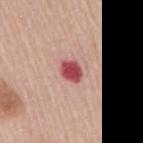<lesion>
<biopsy_status>not biopsied; imaged during a skin examination</biopsy_status>
<patient>
  <sex>female</sex>
  <age_approx>65</age_approx>
</patient>
<image>
  <source>total-body photography crop</source>
  <field_of_view_mm>15</field_of_view_mm>
</image>
<lesion_size>
  <long_diameter_mm_approx>3.0</long_diameter_mm_approx>
</lesion_size>
<automated_metrics>
  <eccentricity>0.7</eccentricity>
  <shape_asymmetry>0.2</shape_asymmetry>
  <cielab_L>52</cielab_L>
  <cielab_a>35</cielab_a>
  <cielab_b>23</cielab_b>
  <vs_skin_darker_L>17.0</vs_skin_darker_L>
  <nevus_likeness_0_100>0</nevus_likeness_0_100>
</automated_metrics>
<site>mid back</site>
<lighting>white-light</lighting>
</lesion>